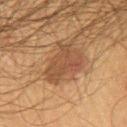* workup — catalogued during a skin exam; not biopsied
* lighting — cross-polarized
* anatomic site — the back
* patient — male, about 65 years old
* imaging modality — 15 mm crop, total-body photography
* size — ≈5 mm